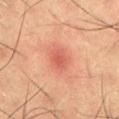– notes: imaged on a skin check; not biopsied